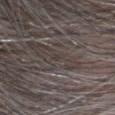The lesion was photographed on a routine skin check and not biopsied; there is no pathology result. The lesion is located on the head or neck. The patient is a male about 60 years old. Cropped from a total-body skin-imaging series; the visible field is about 15 mm.